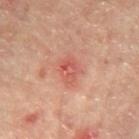Assessment: Recorded during total-body skin imaging; not selected for excision or biopsy. Clinical summary: The lesion is located on the mid back. Imaged with cross-polarized lighting. A lesion tile, about 15 mm wide, cut from a 3D total-body photograph. About 3.5 mm across. A male subject roughly 65 years of age.